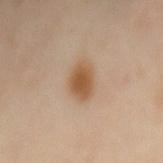Clinical impression: Captured during whole-body skin photography for melanoma surveillance; the lesion was not biopsied. Clinical summary: This image is a 15 mm lesion crop taken from a total-body photograph. The tile uses cross-polarized illumination. The lesion is located on the mid back. Measured at roughly 4.5 mm in maximum diameter. A female patient, aged approximately 40. The total-body-photography lesion software estimated a lesion color around L≈55 a*≈18 b*≈34 in CIELAB and about 11 CIELAB-L* units darker than the surrounding skin.